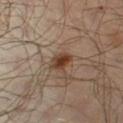The lesion was photographed on a routine skin check and not biopsied; there is no pathology result.
On the leg.
A 15 mm close-up tile from a total-body photography series done for melanoma screening.
Approximately 2.5 mm at its widest.
An algorithmic analysis of the crop reported a classifier nevus-likeness of about 95/100 and lesion-presence confidence of about 100/100.
A male subject approximately 65 years of age.
The tile uses cross-polarized illumination.